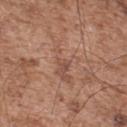Case summary:
* subject: male, in their mid-50s
* image source: total-body-photography crop, ~15 mm field of view
* illumination: white-light
* image-analysis metrics: a footprint of about 9 mm²; a border-irregularity rating of about 9/10, internal color variation of about 2 on a 0–10 scale, and peripheral color asymmetry of about 0.5; a classifier nevus-likeness of about 0/100 and a lesion-detection confidence of about 65/100
* body site: the upper back